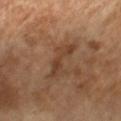Q: Is there a histopathology result?
A: no biopsy performed (imaged during a skin exam)
Q: What lighting was used for the tile?
A: cross-polarized illumination
Q: Patient demographics?
A: female, approximately 70 years of age
Q: What did automated image analysis measure?
A: border irregularity of about 6.5 on a 0–10 scale and radial color variation of about 0.5; a classifier nevus-likeness of about 0/100
Q: How was this image acquired?
A: ~15 mm crop, total-body skin-cancer survey
Q: Lesion size?
A: ~5 mm (longest diameter)
Q: Lesion location?
A: the left forearm